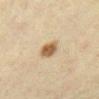Part of a total-body skin-imaging series; this lesion was reviewed on a skin check and was not flagged for biopsy. Located on the right lower leg. A female patient in their 40s. The lesion's longest dimension is about 2.5 mm. Captured under cross-polarized illumination. A 15 mm close-up tile from a total-body photography series done for melanoma screening. Automated image analysis of the tile measured a lesion color around L≈50 a*≈14 b*≈31 in CIELAB, about 13 CIELAB-L* units darker than the surrounding skin, and a lesion-to-skin contrast of about 9.5 (normalized; higher = more distinct). It also reported a within-lesion color-variation index near 3/10 and a peripheral color-asymmetry measure near 1. The software also gave a lesion-detection confidence of about 100/100.